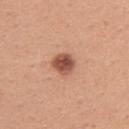The lesion was photographed on a routine skin check and not biopsied; there is no pathology result. The lesion's longest dimension is about 2.5 mm. A female subject, aged around 30. From the right upper arm. This image is a 15 mm lesion crop taken from a total-body photograph.